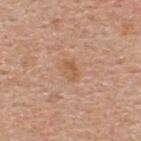Q: Was this lesion biopsied?
A: imaged on a skin check; not biopsied
Q: What did automated image analysis measure?
A: a shape eccentricity near 0.7 and a shape-asymmetry score of about 0.25 (0 = symmetric); a lesion color around L≈57 a*≈21 b*≈34 in CIELAB, roughly 7 lightness units darker than nearby skin, and a normalized lesion–skin contrast near 5.5; a peripheral color-asymmetry measure near 1.5; a lesion-detection confidence of about 100/100
Q: Illumination type?
A: white-light illumination
Q: Lesion size?
A: ~2.5 mm (longest diameter)
Q: Where on the body is the lesion?
A: the upper back
Q: What are the patient's age and sex?
A: male, roughly 55 years of age
Q: What kind of image is this?
A: total-body-photography crop, ~15 mm field of view